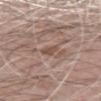The lesion was tiled from a total-body skin photograph and was not biopsied.
Automated image analysis of the tile measured a mean CIELAB color near L≈50 a*≈18 b*≈25, a lesion–skin lightness drop of about 8, and a normalized lesion–skin contrast near 6.5.
A male subject, aged around 70.
Imaged with white-light lighting.
The lesion's longest dimension is about 2.5 mm.
A region of skin cropped from a whole-body photographic capture, roughly 15 mm wide.
The lesion is on the left forearm.